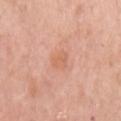The lesion was photographed on a routine skin check and not biopsied; there is no pathology result. Automated tile analysis of the lesion measured an average lesion color of about L≈63 a*≈25 b*≈33 (CIELAB), roughly 7 lightness units darker than nearby skin, and a normalized border contrast of about 5. And it measured a border-irregularity rating of about 3/10, internal color variation of about 1.5 on a 0–10 scale, and radial color variation of about 0.5. Measured at roughly 3 mm in maximum diameter. This is a white-light tile. A female patient about 65 years old. The lesion is on the left upper arm. A close-up tile cropped from a whole-body skin photograph, about 15 mm across.